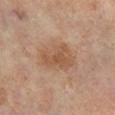biopsy status: no biopsy performed (imaged during a skin exam) | anatomic site: the right lower leg | imaging modality: 15 mm crop, total-body photography | patient: female, about 60 years old.